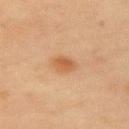biopsy status=no biopsy performed (imaged during a skin exam) | size=≈2.5 mm | lighting=cross-polarized illumination | acquisition=~15 mm tile from a whole-body skin photo | location=the left upper arm | patient=female, about 55 years old.